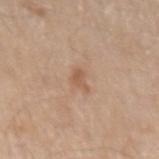The lesion was photographed on a routine skin check and not biopsied; there is no pathology result.
Cropped from a whole-body photographic skin survey; the tile spans about 15 mm.
A male patient, in their 80s.
From the right arm.